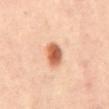Case summary:
- follow-up — imaged on a skin check; not biopsied
- patient — female, in their mid-30s
- site — the front of the torso
- image — 15 mm crop, total-body photography